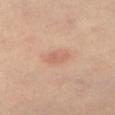Impression:
This lesion was catalogued during total-body skin photography and was not selected for biopsy.
Image and clinical context:
The subject is a female in their mid-60s. This is a cross-polarized tile. The recorded lesion diameter is about 2.5 mm. From the left thigh. A roughly 15 mm field-of-view crop from a total-body skin photograph.